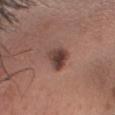The lesion was tiled from a total-body skin photograph and was not biopsied.
A male subject in their mid-30s.
Cropped from a whole-body photographic skin survey; the tile spans about 15 mm.
The lesion is located on the head or neck.
The tile uses white-light illumination.
About 3.5 mm across.